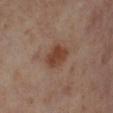This lesion was catalogued during total-body skin photography and was not selected for biopsy.
About 3 mm across.
From the right lower leg.
A female subject, in their mid-50s.
Cropped from a whole-body photographic skin survey; the tile spans about 15 mm.
Captured under cross-polarized illumination.